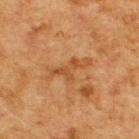<tbp_lesion>
<biopsy_status>not biopsied; imaged during a skin examination</biopsy_status>
<automated_metrics>
  <eccentricity>0.9</eccentricity>
  <shape_asymmetry>0.55</shape_asymmetry>
  <border_irregularity_0_10>7.5</border_irregularity_0_10>
  <color_variation_0_10>1.5</color_variation_0_10>
  <peripheral_color_asymmetry>0.5</peripheral_color_asymmetry>
</automated_metrics>
<lesion_size>
  <long_diameter_mm_approx>5.5</long_diameter_mm_approx>
</lesion_size>
<image>
  <source>total-body photography crop</source>
  <field_of_view_mm>15</field_of_view_mm>
</image>
<lighting>cross-polarized</lighting>
<patient>
  <sex>male</sex>
  <age_approx>75</age_approx>
</patient>
<site>back</site>
</tbp_lesion>